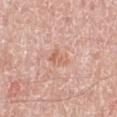Clinical impression: No biopsy was performed on this lesion — it was imaged during a full skin examination and was not determined to be concerning.